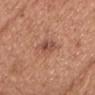No biopsy was performed on this lesion — it was imaged during a full skin examination and was not determined to be concerning. Automated tile analysis of the lesion measured an eccentricity of roughly 0.85 and a shape-asymmetry score of about 0.2 (0 = symmetric). A female subject, about 40 years old. The lesion is located on the head or neck. The tile uses white-light illumination. A 15 mm close-up extracted from a 3D total-body photography capture.